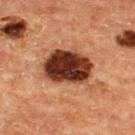Clinical impression:
The lesion was photographed on a routine skin check and not biopsied; there is no pathology result.
Clinical summary:
This is a cross-polarized tile. A male subject, about 65 years old. The lesion is on the upper back. The recorded lesion diameter is about 6 mm. A lesion tile, about 15 mm wide, cut from a 3D total-body photograph. The total-body-photography lesion software estimated a lesion color around L≈33 a*≈24 b*≈29 in CIELAB, roughly 22 lightness units darker than nearby skin, and a normalized border contrast of about 17. It also reported a color-variation rating of about 6.5/10.